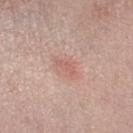  biopsy_status: not biopsied; imaged during a skin examination
  lighting: white-light
  image:
    source: total-body photography crop
    field_of_view_mm: 15
  patient:
    sex: female
    age_approx: 50
  site: leg